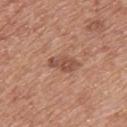The lesion was photographed on a routine skin check and not biopsied; there is no pathology result.